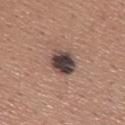Q: Is there a histopathology result?
A: catalogued during a skin exam; not biopsied
Q: Automated lesion metrics?
A: an outline eccentricity of about 0.7 (0 = round, 1 = elongated) and a symmetry-axis asymmetry near 0.15; a lesion color around L≈41 a*≈14 b*≈18 in CIELAB and roughly 18 lightness units darker than nearby skin; border irregularity of about 1.5 on a 0–10 scale, a within-lesion color-variation index near 7/10, and peripheral color asymmetry of about 2; a lesion-detection confidence of about 100/100
Q: How was this image acquired?
A: total-body-photography crop, ~15 mm field of view
Q: What lighting was used for the tile?
A: white-light
Q: Where on the body is the lesion?
A: the upper back
Q: Patient demographics?
A: male, aged 43–47